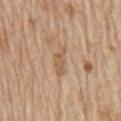workup = imaged on a skin check; not biopsied
illumination = white-light
patient = male, aged 68–72
acquisition = ~15 mm tile from a whole-body skin photo
location = the mid back